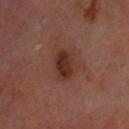{"biopsy_status": "not biopsied; imaged during a skin examination", "site": "head or neck", "lighting": "cross-polarized", "patient": {"age_approx": 65}, "image": {"source": "total-body photography crop", "field_of_view_mm": 15}}